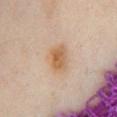notes = total-body-photography surveillance lesion; no biopsy
subject = female, aged approximately 40
acquisition = ~15 mm tile from a whole-body skin photo
lesion size = ≈4 mm
site = the chest
image-analysis metrics = a lesion-detection confidence of about 100/100
lighting = cross-polarized